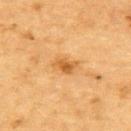The lesion was photographed on a routine skin check and not biopsied; there is no pathology result. Approximately 3 mm at its widest. A 15 mm close-up extracted from a 3D total-body photography capture. This is a cross-polarized tile. From the upper back. Automated tile analysis of the lesion measured about 10 CIELAB-L* units darker than the surrounding skin and a lesion-to-skin contrast of about 7.5 (normalized; higher = more distinct). It also reported a border-irregularity rating of about 2.5/10 and radial color variation of about 1. The patient is a male aged 83 or over.